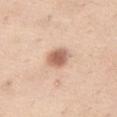Captured during whole-body skin photography for melanoma surveillance; the lesion was not biopsied. Measured at roughly 3 mm in maximum diameter. On the right thigh. Imaged with white-light lighting. A female patient, aged 53 to 57. A 15 mm close-up tile from a total-body photography series done for melanoma screening.